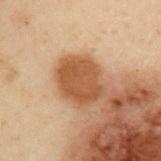Part of a total-body skin-imaging series; this lesion was reviewed on a skin check and was not flagged for biopsy.
A 15 mm close-up extracted from a 3D total-body photography capture.
From the left upper arm.
Approximately 4.5 mm at its widest.
Imaged with cross-polarized lighting.
A male patient in their mid-50s.